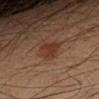Recorded during total-body skin imaging; not selected for excision or biopsy.
A roughly 15 mm field-of-view crop from a total-body skin photograph.
The tile uses cross-polarized illumination.
A male subject aged 33 to 37.
Automated image analysis of the tile measured an area of roughly 6 mm² and a symmetry-axis asymmetry near 0.3. The analysis additionally found a lesion-detection confidence of about 100/100.
The lesion's longest dimension is about 3 mm.
Located on the left forearm.